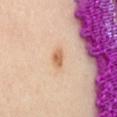biopsy_status: not biopsied; imaged during a skin examination
image:
  source: total-body photography crop
  field_of_view_mm: 15
patient:
  sex: female
  age_approx: 40
site: left thigh